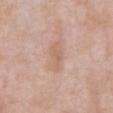notes: catalogued during a skin exam; not biopsied
patient: male, about 55 years old
image-analysis metrics: a footprint of about 6.5 mm², an outline eccentricity of about 0.85 (0 = round, 1 = elongated), and a shape-asymmetry score of about 0.25 (0 = symmetric); a lesion color around L≈63 a*≈19 b*≈29 in CIELAB; a border-irregularity index near 2.5/10, internal color variation of about 1.5 on a 0–10 scale, and radial color variation of about 0.5
image source: 15 mm crop, total-body photography
location: the abdomen
illumination: white-light illumination
diameter: about 4 mm